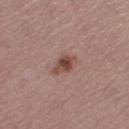Image and clinical context:
From the right thigh. A female patient, aged approximately 55. Imaged with white-light lighting. About 3.5 mm across. This image is a 15 mm lesion crop taken from a total-body photograph.